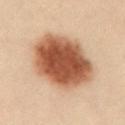Assessment:
No biopsy was performed on this lesion — it was imaged during a full skin examination and was not determined to be concerning.
Context:
On the abdomen. This is a cross-polarized tile. A female patient about 30 years old. A close-up tile cropped from a whole-body skin photograph, about 15 mm across. The lesion-visualizer software estimated a mean CIELAB color near L≈42 a*≈20 b*≈28. And it measured a border-irregularity rating of about 1.5/10 and a color-variation rating of about 5/10. And it measured an automated nevus-likeness rating near 100 out of 100 and a lesion-detection confidence of about 100/100. The lesion's longest dimension is about 7.5 mm.